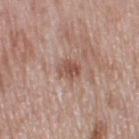Assessment:
No biopsy was performed on this lesion — it was imaged during a full skin examination and was not determined to be concerning.
Acquisition and patient details:
From the right thigh. Approximately 2.5 mm at its widest. A female patient in their 50s. Captured under white-light illumination. A roughly 15 mm field-of-view crop from a total-body skin photograph.